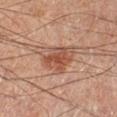Assessment:
Captured during whole-body skin photography for melanoma surveillance; the lesion was not biopsied.
Clinical summary:
A 15 mm crop from a total-body photograph taken for skin-cancer surveillance. Approximately 4.5 mm at its widest. The lesion-visualizer software estimated a footprint of about 12 mm², an eccentricity of roughly 0.55, and a symmetry-axis asymmetry near 0.3. The software also gave a lesion color around L≈46 a*≈21 b*≈27 in CIELAB, about 9 CIELAB-L* units darker than the surrounding skin, and a normalized lesion–skin contrast near 7. From the right lower leg. A male subject approximately 50 years of age. The tile uses cross-polarized illumination.